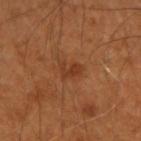{"biopsy_status": "not biopsied; imaged during a skin examination", "automated_metrics": {"eccentricity": 0.75, "shape_asymmetry": 0.35, "vs_skin_contrast_norm": 6.0, "border_irregularity_0_10": 4.0, "color_variation_0_10": 1.0, "peripheral_color_asymmetry": 0.5, "nevus_likeness_0_100": 5, "lesion_detection_confidence_0_100": 100}, "patient": {"sex": "male", "age_approx": 50}, "image": {"source": "total-body photography crop", "field_of_view_mm": 15}, "site": "left arm", "lighting": "cross-polarized", "lesion_size": {"long_diameter_mm_approx": 2.5}}